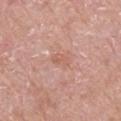Findings:
– follow-up — catalogued during a skin exam; not biopsied
– size — about 2.5 mm
– anatomic site — the right upper arm
– subject — male, roughly 65 years of age
– lighting — white-light illumination
– image — ~15 mm tile from a whole-body skin photo